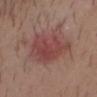Clinical impression: The lesion was photographed on a routine skin check and not biopsied; there is no pathology result. Image and clinical context: This is a white-light tile. A male patient in their 30s. From the head or neck. About 6 mm across. A roughly 15 mm field-of-view crop from a total-body skin photograph.